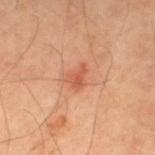Notes:
• follow-up: imaged on a skin check; not biopsied
• anatomic site: the left lower leg
• illumination: cross-polarized
• diameter: ≈2.5 mm
• patient: male, aged around 70
• acquisition: total-body-photography crop, ~15 mm field of view